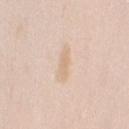lighting — white-light illumination | automated metrics — a footprint of about 3.5 mm² and a shape eccentricity near 0.95; an average lesion color of about L≈73 a*≈15 b*≈32 (CIELAB) and roughly 6 lightness units darker than nearby skin; a border-irregularity rating of about 4/10, internal color variation of about 0 on a 0–10 scale, and radial color variation of about 0 | image source — total-body-photography crop, ~15 mm field of view | lesion diameter — ~3.5 mm (longest diameter) | location — the right upper arm | patient — female, about 25 years old.